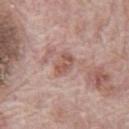– biopsy status — total-body-photography surveillance lesion; no biopsy
– location — the abdomen
– illumination — white-light illumination
– automated metrics — roughly 10 lightness units darker than nearby skin and a normalized border contrast of about 7; border irregularity of about 3 on a 0–10 scale and a color-variation rating of about 3/10; a nevus-likeness score of about 0/100 and a detector confidence of about 100 out of 100 that the crop contains a lesion
– subject — male, aged 68–72
– image — total-body-photography crop, ~15 mm field of view